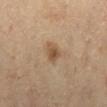No biopsy was performed on this lesion — it was imaged during a full skin examination and was not determined to be concerning. A subject in their 60s. Longest diameter approximately 2.5 mm. Automated image analysis of the tile measured a lesion color around L≈51 a*≈16 b*≈32 in CIELAB, a lesion–skin lightness drop of about 10, and a lesion-to-skin contrast of about 7.5 (normalized; higher = more distinct). It also reported a border-irregularity rating of about 2.5/10 and internal color variation of about 3 on a 0–10 scale. This is a cross-polarized tile. A roughly 15 mm field-of-view crop from a total-body skin photograph. The lesion is located on the right lower leg.